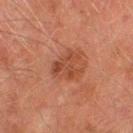This lesion was catalogued during total-body skin photography and was not selected for biopsy. On the left thigh. Approximately 4 mm at its widest. The tile uses cross-polarized illumination. An algorithmic analysis of the crop reported a lesion area of about 7 mm², a shape eccentricity near 0.7, and a shape-asymmetry score of about 0.55 (0 = symmetric). The analysis additionally found about 7 CIELAB-L* units darker than the surrounding skin and a lesion-to-skin contrast of about 6 (normalized; higher = more distinct). Cropped from a whole-body photographic skin survey; the tile spans about 15 mm. A male patient, roughly 75 years of age.